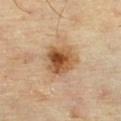  biopsy_status: not biopsied; imaged during a skin examination
  image:
    source: total-body photography crop
    field_of_view_mm: 15
  patient:
    sex: male
    age_approx: 70
  automated_metrics:
    area_mm2_approx: 13.0
    eccentricity: 0.65
    shape_asymmetry: 0.25
    border_irregularity_0_10: 2.5
    peripheral_color_asymmetry: 3.0
    nevus_likeness_0_100: 95
  site: right thigh